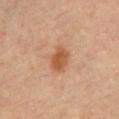biopsy status: total-body-photography surveillance lesion; no biopsy
TBP lesion metrics: an area of roughly 6.5 mm², an eccentricity of roughly 0.7, and two-axis asymmetry of about 0.25; a mean CIELAB color near L≈53 a*≈23 b*≈36 and a normalized lesion–skin contrast near 8; border irregularity of about 2 on a 0–10 scale and a peripheral color-asymmetry measure near 1
image: ~15 mm crop, total-body skin-cancer survey
illumination: cross-polarized
subject: female, in their mid- to late 40s
location: the chest
size: ≈3.5 mm